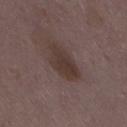Captured during whole-body skin photography for melanoma surveillance; the lesion was not biopsied.
On the lower back.
The tile uses white-light illumination.
A close-up tile cropped from a whole-body skin photograph, about 15 mm across.
Longest diameter approximately 5 mm.
The patient is a female in their mid- to late 30s.